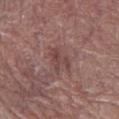Imaged during a routine full-body skin examination; the lesion was not biopsied and no histopathology is available.
The tile uses white-light illumination.
Located on the left lower leg.
A roughly 15 mm field-of-view crop from a total-body skin photograph.
A female patient, aged approximately 80.
Longest diameter approximately 3 mm.